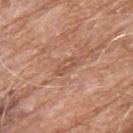The patient is a male aged 78 to 82. Cropped from a whole-body photographic skin survey; the tile spans about 15 mm. The lesion is on the upper back.